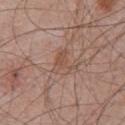Notes:
• notes — total-body-photography surveillance lesion; no biopsy
• patient — male, aged 68 to 72
• anatomic site — the chest
• image source — total-body-photography crop, ~15 mm field of view